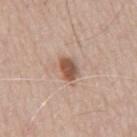The lesion was tiled from a total-body skin photograph and was not biopsied. Automated tile analysis of the lesion measured a lesion area of about 4.5 mm² and a symmetry-axis asymmetry near 0.15. The software also gave a border-irregularity rating of about 1.5/10, a color-variation rating of about 2.5/10, and radial color variation of about 1. The software also gave an automated nevus-likeness rating near 90 out of 100 and a lesion-detection confidence of about 100/100. Located on the mid back. The subject is a male in their mid- to late 60s. A roughly 15 mm field-of-view crop from a total-body skin photograph.